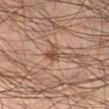This lesion was catalogued during total-body skin photography and was not selected for biopsy.
An algorithmic analysis of the crop reported a lesion area of about 4 mm² and a shape-asymmetry score of about 0.45 (0 = symmetric). It also reported a classifier nevus-likeness of about 90/100 and a detector confidence of about 100 out of 100 that the crop contains a lesion.
The lesion's longest dimension is about 3.5 mm.
Cropped from a whole-body photographic skin survey; the tile spans about 15 mm.
A male subject, aged 43 to 47.
From the right lower leg.
The tile uses cross-polarized illumination.